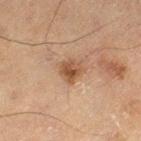Impression:
The lesion was tiled from a total-body skin photograph and was not biopsied.
Acquisition and patient details:
A 15 mm crop from a total-body photograph taken for skin-cancer surveillance. Measured at roughly 2.5 mm in maximum diameter. Located on the left thigh. The tile uses cross-polarized illumination. A male subject, aged around 70.